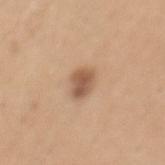Impression: Recorded during total-body skin imaging; not selected for excision or biopsy. Context: The lesion is on the mid back. The patient is a male aged 48–52. A lesion tile, about 15 mm wide, cut from a 3D total-body photograph. This is a white-light tile. Automated image analysis of the tile measured a footprint of about 5.5 mm², a shape eccentricity near 0.65, and two-axis asymmetry of about 0.2. And it measured a lesion color around L≈56 a*≈19 b*≈32 in CIELAB, a lesion–skin lightness drop of about 12, and a lesion-to-skin contrast of about 8 (normalized; higher = more distinct).